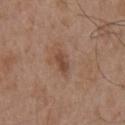Captured during whole-body skin photography for melanoma surveillance; the lesion was not biopsied. From the chest. A roughly 15 mm field-of-view crop from a total-body skin photograph. The tile uses white-light illumination. The lesion's longest dimension is about 2.5 mm. A male patient, aged 53–57. An algorithmic analysis of the crop reported a footprint of about 3.5 mm², a shape eccentricity near 0.85, and a shape-asymmetry score of about 0.25 (0 = symmetric). The software also gave about 9 CIELAB-L* units darker than the surrounding skin and a normalized lesion–skin contrast near 7. And it measured a border-irregularity rating of about 2.5/10 and a color-variation rating of about 1/10. The analysis additionally found a nevus-likeness score of about 5/100.